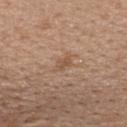Findings:
• notes · catalogued during a skin exam; not biopsied